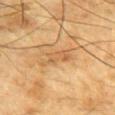No biopsy was performed on this lesion — it was imaged during a full skin examination and was not determined to be concerning.